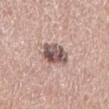{
  "biopsy_status": "not biopsied; imaged during a skin examination",
  "lighting": "white-light",
  "site": "left lower leg",
  "automated_metrics": {
    "cielab_L": 54,
    "cielab_a": 17,
    "cielab_b": 20,
    "vs_skin_darker_L": 15.0,
    "vs_skin_contrast_norm": 10.0,
    "nevus_likeness_0_100": 10,
    "lesion_detection_confidence_0_100": 100
  },
  "image": {
    "source": "total-body photography crop",
    "field_of_view_mm": 15
  },
  "lesion_size": {
    "long_diameter_mm_approx": 4.0
  },
  "patient": {
    "sex": "male",
    "age_approx": 60
  }
}